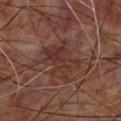A male subject, aged 58 to 62. Captured under cross-polarized illumination. The total-body-photography lesion software estimated border irregularity of about 6 on a 0–10 scale, internal color variation of about 4 on a 0–10 scale, and radial color variation of about 1.5. The software also gave a nevus-likeness score of about 0/100 and a lesion-detection confidence of about 85/100. Located on the upper back. This image is a 15 mm lesion crop taken from a total-body photograph.